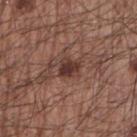Impression: Part of a total-body skin-imaging series; this lesion was reviewed on a skin check and was not flagged for biopsy. Background: Located on the right upper arm. The tile uses white-light illumination. Longest diameter approximately 2.5 mm. Cropped from a whole-body photographic skin survey; the tile spans about 15 mm. The lesion-visualizer software estimated a nevus-likeness score of about 75/100 and a lesion-detection confidence of about 100/100. The patient is a male about 65 years old.